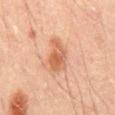follow-up: total-body-photography surveillance lesion; no biopsy | lesion diameter: ~4 mm (longest diameter) | image source: ~15 mm tile from a whole-body skin photo | automated metrics: a mean CIELAB color near L≈50 a*≈22 b*≈31, about 9 CIELAB-L* units darker than the surrounding skin, and a normalized lesion–skin contrast near 7.5; a border-irregularity index near 3.5/10, internal color variation of about 4.5 on a 0–10 scale, and a peripheral color-asymmetry measure near 1.5 | anatomic site: the abdomen | patient: male, approximately 45 years of age.